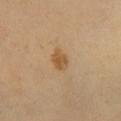notes: total-body-photography surveillance lesion; no biopsy | image source: 15 mm crop, total-body photography | illumination: cross-polarized | subject: approximately 70 years of age | lesion diameter: about 3 mm | site: the right lower leg.